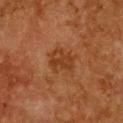The lesion was photographed on a routine skin check and not biopsied; there is no pathology result. About 3.5 mm across. The tile uses cross-polarized illumination. A female patient aged 48 to 52. On the chest. Automated image analysis of the tile measured a footprint of about 7.5 mm² and a shape-asymmetry score of about 0.3 (0 = symmetric). The analysis additionally found an average lesion color of about L≈32 a*≈21 b*≈31 (CIELAB), about 6 CIELAB-L* units darker than the surrounding skin, and a lesion-to-skin contrast of about 6.5 (normalized; higher = more distinct). It also reported a border-irregularity index near 3/10. The analysis additionally found a classifier nevus-likeness of about 5/100 and a detector confidence of about 100 out of 100 that the crop contains a lesion. A region of skin cropped from a whole-body photographic capture, roughly 15 mm wide.